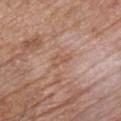Q: What is the anatomic site?
A: the chest
Q: What kind of image is this?
A: total-body-photography crop, ~15 mm field of view
Q: What lighting was used for the tile?
A: white-light
Q: Who is the patient?
A: male, roughly 55 years of age